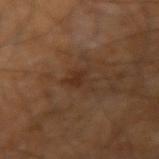follow-up: imaged on a skin check; not biopsied
lighting: cross-polarized
subject: male, aged 58 to 62
site: the right arm
imaging modality: ~15 mm tile from a whole-body skin photo
diameter: ≈3 mm